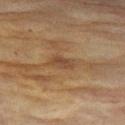Part of a total-body skin-imaging series; this lesion was reviewed on a skin check and was not flagged for biopsy. A roughly 15 mm field-of-view crop from a total-body skin photograph. The recorded lesion diameter is about 3.5 mm. The lesion is located on the left thigh. The lesion-visualizer software estimated a lesion color around L≈39 a*≈16 b*≈28 in CIELAB, a lesion–skin lightness drop of about 7, and a lesion-to-skin contrast of about 6 (normalized; higher = more distinct). The tile uses cross-polarized illumination. A female patient, aged around 80.